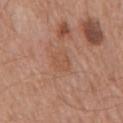No biopsy was performed on this lesion — it was imaged during a full skin examination and was not determined to be concerning.
A 15 mm crop from a total-body photograph taken for skin-cancer surveillance.
Located on the chest.
A male subject, aged approximately 65.